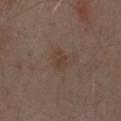* biopsy status — imaged on a skin check; not biopsied
* subject — male, in their 70s
* acquisition — total-body-photography crop, ~15 mm field of view
* diameter — ~2.5 mm (longest diameter)
* location — the front of the torso
* tile lighting — white-light illumination